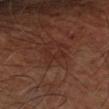Findings:
* workup — imaged on a skin check; not biopsied
* subject — roughly 65 years of age
* diameter — ≈3 mm
* image-analysis metrics — a border-irregularity rating of about 6.5/10 and a within-lesion color-variation index near 0/10
* anatomic site — the left forearm
* imaging modality — ~15 mm crop, total-body skin-cancer survey